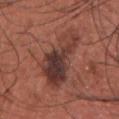Notes:
- follow-up · imaged on a skin check; not biopsied
- tile lighting · white-light illumination
- location · the chest
- subject · male, aged 33 to 37
- lesion diameter · about 7 mm
- acquisition · 15 mm crop, total-body photography